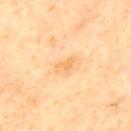follow-up = imaged on a skin check; not biopsied
subject = male, in their mid-50s
imaging modality = ~15 mm tile from a whole-body skin photo
automated lesion analysis = a footprint of about 3 mm² and a symmetry-axis asymmetry near 0.4; roughly 7 lightness units darker than nearby skin and a normalized lesion–skin contrast near 5.5; a classifier nevus-likeness of about 0/100 and a detector confidence of about 100 out of 100 that the crop contains a lesion
site = the mid back